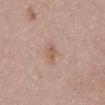workup: total-body-photography surveillance lesion; no biopsy | lesion diameter: ≈2.5 mm | TBP lesion metrics: a footprint of about 2.5 mm², a shape eccentricity near 0.9, and two-axis asymmetry of about 0.35; a within-lesion color-variation index near 0/10 and a peripheral color-asymmetry measure near 0 | image source: total-body-photography crop, ~15 mm field of view | subject: female, aged 28–32 | body site: the abdomen.